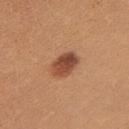No biopsy was performed on this lesion — it was imaged during a full skin examination and was not determined to be concerning.
The total-body-photography lesion software estimated an average lesion color of about L≈48 a*≈25 b*≈32 (CIELAB), about 14 CIELAB-L* units darker than the surrounding skin, and a normalized lesion–skin contrast near 10. And it measured a border-irregularity rating of about 1.5/10, a within-lesion color-variation index near 6/10, and peripheral color asymmetry of about 2. The software also gave a nevus-likeness score of about 95/100 and a detector confidence of about 100 out of 100 that the crop contains a lesion.
This image is a 15 mm lesion crop taken from a total-body photograph.
Longest diameter approximately 4 mm.
Imaged with white-light lighting.
A female subject about 25 years old.
The lesion is on the left upper arm.